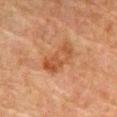follow-up = catalogued during a skin exam; not biopsied
image source = 15 mm crop, total-body photography
patient = male, aged 78–82
lighting = cross-polarized
site = the abdomen